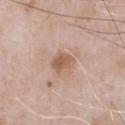Image and clinical context:
The lesion's longest dimension is about 2.5 mm. This is a white-light tile. The patient is a male aged 48–52. The lesion is on the chest. A 15 mm crop from a total-body photograph taken for skin-cancer surveillance.